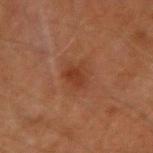Measured at roughly 2.5 mm in maximum diameter. A male subject, roughly 65 years of age. Imaged with cross-polarized lighting. An algorithmic analysis of the crop reported a normalized lesion–skin contrast near 6.5. And it measured an automated nevus-likeness rating near 35 out of 100 and a lesion-detection confidence of about 100/100. The lesion is on the right upper arm. A region of skin cropped from a whole-body photographic capture, roughly 15 mm wide.